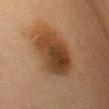follow-up — no biopsy performed (imaged during a skin exam) | imaging modality — total-body-photography crop, ~15 mm field of view | subject — female, aged around 55 | tile lighting — cross-polarized illumination | lesion diameter — ~7.5 mm (longest diameter) | location — the front of the torso.